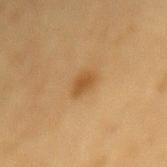| key | value |
|---|---|
| biopsy status | no biopsy performed (imaged during a skin exam) |
| tile lighting | cross-polarized illumination |
| patient | male, aged approximately 85 or older |
| location | the mid back |
| image source | total-body-photography crop, ~15 mm field of view |